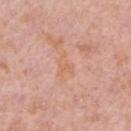Assessment:
Part of a total-body skin-imaging series; this lesion was reviewed on a skin check and was not flagged for biopsy.
Background:
From the left lower leg. This is a white-light tile. Automated image analysis of the tile measured a shape eccentricity near 0.75 and a shape-asymmetry score of about 0.5 (0 = symmetric). The analysis additionally found a normalized border contrast of about 5. A female subject, about 40 years old. A roughly 15 mm field-of-view crop from a total-body skin photograph.